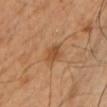Case summary:
• biopsy status — imaged on a skin check; not biopsied
• patient — male, about 40 years old
• anatomic site — the chest
• acquisition — ~15 mm crop, total-body skin-cancer survey